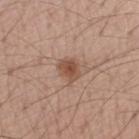notes — no biopsy performed (imaged during a skin exam); anatomic site — the right forearm; imaging modality — ~15 mm crop, total-body skin-cancer survey; tile lighting — white-light; patient — male, aged around 55; TBP lesion metrics — a footprint of about 5 mm², a shape eccentricity near 0.65, and two-axis asymmetry of about 0.25.